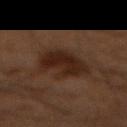{"biopsy_status": "not biopsied; imaged during a skin examination", "patient": {"sex": "male", "age_approx": 60}, "automated_metrics": {"cielab_L": 21, "cielab_a": 16, "cielab_b": 21, "vs_skin_darker_L": 8.0, "vs_skin_contrast_norm": 9.5, "border_irregularity_0_10": 3.5, "color_variation_0_10": 3.0, "peripheral_color_asymmetry": 1.0}, "site": "back", "image": {"source": "total-body photography crop", "field_of_view_mm": 15}, "lesion_size": {"long_diameter_mm_approx": 5.0}, "lighting": "cross-polarized"}